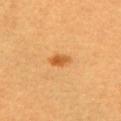Q: Was a biopsy performed?
A: catalogued during a skin exam; not biopsied